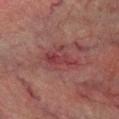<case>
  <biopsy_status>not biopsied; imaged during a skin examination</biopsy_status>
  <site>right lower leg</site>
  <patient>
    <sex>male</sex>
    <age_approx>75</age_approx>
  </patient>
  <lesion_size>
    <long_diameter_mm_approx>4.5</long_diameter_mm_approx>
  </lesion_size>
  <lighting>cross-polarized</lighting>
  <image>
    <source>total-body photography crop</source>
    <field_of_view_mm>15</field_of_view_mm>
  </image>
  <automated_metrics>
    <area_mm2_approx>6.0</area_mm2_approx>
    <eccentricity>0.9</eccentricity>
    <shape_asymmetry>0.35</shape_asymmetry>
    <vs_skin_darker_L>7.0</vs_skin_darker_L>
    <vs_skin_contrast_norm>6.5</vs_skin_contrast_norm>
    <border_irregularity_0_10>5.0</border_irregularity_0_10>
    <color_variation_0_10>2.5</color_variation_0_10>
    <peripheral_color_asymmetry>1.0</peripheral_color_asymmetry>
    <nevus_likeness_0_100>0</nevus_likeness_0_100>
    <lesion_detection_confidence_0_100>95</lesion_detection_confidence_0_100>
  </automated_metrics>
</case>